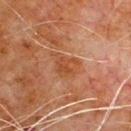Findings:
* notes: total-body-photography surveillance lesion; no biopsy
* lighting: cross-polarized illumination
* lesion size: ≈4 mm
* anatomic site: the chest
* imaging modality: 15 mm crop, total-body photography
* patient: male, roughly 80 years of age